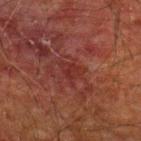The lesion was tiled from a total-body skin photograph and was not biopsied.
The recorded lesion diameter is about 3 mm.
Captured under cross-polarized illumination.
A 15 mm close-up tile from a total-body photography series done for melanoma screening.
A male subject, aged approximately 60.
On the back.